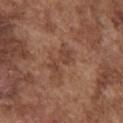The lesion was photographed on a routine skin check and not biopsied; there is no pathology result. Located on the chest. Captured under white-light illumination. Cropped from a whole-body photographic skin survey; the tile spans about 15 mm. A male patient aged 73–77. About 4 mm across. Automated tile analysis of the lesion measured an average lesion color of about L≈43 a*≈21 b*≈29 (CIELAB). It also reported a border-irregularity index near 7.5/10, internal color variation of about 1 on a 0–10 scale, and peripheral color asymmetry of about 0.5. And it measured a detector confidence of about 100 out of 100 that the crop contains a lesion.